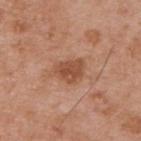Image and clinical context:
The subject is a male aged 53 to 57. The lesion's longest dimension is about 3.5 mm. Imaged with white-light lighting. This image is a 15 mm lesion crop taken from a total-body photograph. On the upper back.